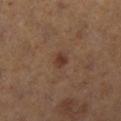No biopsy was performed on this lesion — it was imaged during a full skin examination and was not determined to be concerning.
About 2 mm across.
The tile uses cross-polarized illumination.
From the right lower leg.
Cropped from a whole-body photographic skin survey; the tile spans about 15 mm.
The patient is a female approximately 40 years of age.